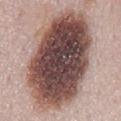lesion diameter = ~14 mm (longest diameter)
anatomic site = the mid back
TBP lesion metrics = a border-irregularity rating of about 2/10 and peripheral color asymmetry of about 3; a nevus-likeness score of about 95/100 and a lesion-detection confidence of about 100/100
image source = ~15 mm crop, total-body skin-cancer survey
tile lighting = white-light illumination
patient = female, aged approximately 50